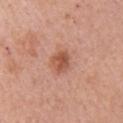Clinical impression:
The lesion was photographed on a routine skin check and not biopsied; there is no pathology result.
Background:
Cropped from a total-body skin-imaging series; the visible field is about 15 mm. From the right upper arm. A female patient, about 60 years old.